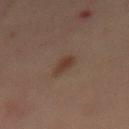<tbp_lesion>
  <biopsy_status>not biopsied; imaged during a skin examination</biopsy_status>
  <lesion_size>
    <long_diameter_mm_approx>3.0</long_diameter_mm_approx>
  </lesion_size>
  <lighting>cross-polarized</lighting>
  <patient>
    <sex>male</sex>
    <age_approx>40</age_approx>
  </patient>
  <site>mid back</site>
  <image>
    <source>total-body photography crop</source>
    <field_of_view_mm>15</field_of_view_mm>
  </image>
</tbp_lesion>